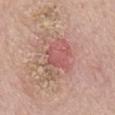biopsy status=no biopsy performed (imaged during a skin exam)